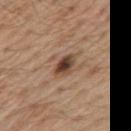| feature | finding |
|---|---|
| workup | imaged on a skin check; not biopsied |
| lesion size | about 3 mm |
| TBP lesion metrics | a lesion color around L≈43 a*≈18 b*≈28 in CIELAB, roughly 14 lightness units darker than nearby skin, and a normalized lesion–skin contrast near 11; border irregularity of about 2 on a 0–10 scale and peripheral color asymmetry of about 1.5 |
| location | the chest |
| acquisition | ~15 mm tile from a whole-body skin photo |
| patient | male, aged around 70 |